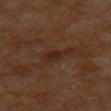Part of a total-body skin-imaging series; this lesion was reviewed on a skin check and was not flagged for biopsy.